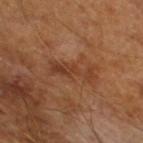This lesion was catalogued during total-body skin photography and was not selected for biopsy.
This is a cross-polarized tile.
A male subject, aged 63 to 67.
The lesion-visualizer software estimated an average lesion color of about L≈38 a*≈22 b*≈32 (CIELAB), a lesion–skin lightness drop of about 7, and a normalized lesion–skin contrast near 6.
A lesion tile, about 15 mm wide, cut from a 3D total-body photograph.